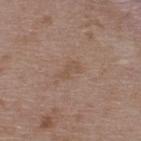* follow-up: catalogued during a skin exam; not biopsied
* body site: the upper back
* image source: 15 mm crop, total-body photography
* lesion diameter: about 2.5 mm
* lighting: white-light illumination
* subject: male, aged approximately 50